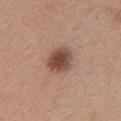This lesion was catalogued during total-body skin photography and was not selected for biopsy. The lesion is on the upper back. A 15 mm crop from a total-body photograph taken for skin-cancer surveillance. Measured at roughly 3.5 mm in maximum diameter. Captured under white-light illumination. The subject is a female aged approximately 40.